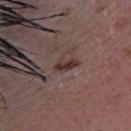Clinical impression:
Recorded during total-body skin imaging; not selected for excision or biopsy.
Image and clinical context:
A lesion tile, about 15 mm wide, cut from a 3D total-body photograph. The patient is a female about 50 years old. The lesion's longest dimension is about 3 mm. The tile uses white-light illumination. On the head or neck. Automated tile analysis of the lesion measured a footprint of about 3.5 mm² and a shape eccentricity near 0.9. The software also gave peripheral color asymmetry of about 0.5. The analysis additionally found a classifier nevus-likeness of about 20/100.